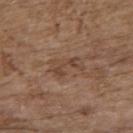Clinical impression:
This lesion was catalogued during total-body skin photography and was not selected for biopsy.
Clinical summary:
From the upper back. A 15 mm close-up extracted from a 3D total-body photography capture. The patient is a female aged around 65. About 4 mm across. The tile uses white-light illumination. Automated tile analysis of the lesion measured a border-irregularity rating of about 7/10, a within-lesion color-variation index near 1.5/10, and peripheral color asymmetry of about 0.5.